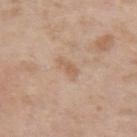Notes:
– workup: catalogued during a skin exam; not biopsied
– automated metrics: a mean CIELAB color near L≈60 a*≈17 b*≈31 and a lesion-to-skin contrast of about 5.5 (normalized; higher = more distinct); a border-irregularity rating of about 3.5/10, a color-variation rating of about 1.5/10, and radial color variation of about 0.5; a classifier nevus-likeness of about 0/100 and a detector confidence of about 100 out of 100 that the crop contains a lesion
– diameter: ≈3 mm
– subject: male, approximately 60 years of age
– acquisition: ~15 mm crop, total-body skin-cancer survey
– location: the arm
– illumination: white-light